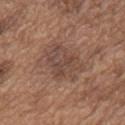workup: catalogued during a skin exam; not biopsied | subject: male, aged around 75 | acquisition: ~15 mm crop, total-body skin-cancer survey | site: the back.